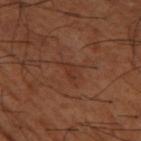Assessment: Captured during whole-body skin photography for melanoma surveillance; the lesion was not biopsied. Background: The patient is a male approximately 65 years of age. A lesion tile, about 15 mm wide, cut from a 3D total-body photograph. Captured under cross-polarized illumination. The lesion is on the left thigh. The lesion's longest dimension is about 2.5 mm.